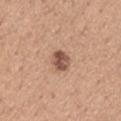The lesion was photographed on a routine skin check and not biopsied; there is no pathology result.